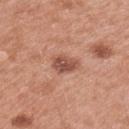Assessment: Recorded during total-body skin imaging; not selected for excision or biopsy. Clinical summary: On the left upper arm. The subject is a male about 55 years old. Cropped from a whole-body photographic skin survey; the tile spans about 15 mm. The recorded lesion diameter is about 3 mm. Imaged with white-light lighting.